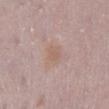Impression: Recorded during total-body skin imaging; not selected for excision or biopsy. Acquisition and patient details: The lesion is on the right lower leg. A lesion tile, about 15 mm wide, cut from a 3D total-body photograph. About 3 mm across. The subject is a female in their 30s.